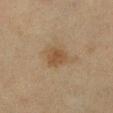The lesion was photographed on a routine skin check and not biopsied; there is no pathology result.